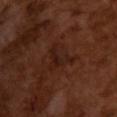Imaged during a routine full-body skin examination; the lesion was not biopsied and no histopathology is available.
The patient is a male aged approximately 65.
The lesion's longest dimension is about 4.5 mm.
A roughly 15 mm field-of-view crop from a total-body skin photograph.
Imaged with cross-polarized lighting.